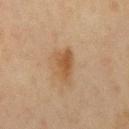The lesion was tiled from a total-body skin photograph and was not biopsied. Located on the right upper arm. Cropped from a whole-body photographic skin survey; the tile spans about 15 mm. Captured under cross-polarized illumination. The lesion-visualizer software estimated a border-irregularity rating of about 3.5/10 and internal color variation of about 3.5 on a 0–10 scale. The subject is a female aged around 45. Approximately 3.5 mm at its widest.